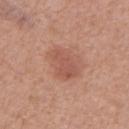Recorded during total-body skin imaging; not selected for excision or biopsy. Cropped from a total-body skin-imaging series; the visible field is about 15 mm. A male subject about 50 years old. On the right upper arm. Automated image analysis of the tile measured an average lesion color of about L≈54 a*≈25 b*≈28 (CIELAB) and a lesion–skin lightness drop of about 8. The analysis additionally found a nevus-likeness score of about 55/100 and a detector confidence of about 100 out of 100 that the crop contains a lesion. Captured under white-light illumination. About 4 mm across.